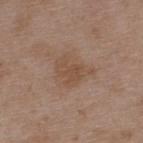Part of a total-body skin-imaging series; this lesion was reviewed on a skin check and was not flagged for biopsy. The lesion is on the upper back. A 15 mm crop from a total-body photograph taken for skin-cancer surveillance. The subject is a male about 50 years old. The lesion's longest dimension is about 4 mm.